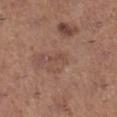Q: Is there a histopathology result?
A: imaged on a skin check; not biopsied
Q: What did automated image analysis measure?
A: a footprint of about 2.5 mm² and two-axis asymmetry of about 0.4; a classifier nevus-likeness of about 0/100 and lesion-presence confidence of about 100/100
Q: What is the lesion's diameter?
A: ≈2.5 mm
Q: What is the imaging modality?
A: ~15 mm tile from a whole-body skin photo
Q: Who is the patient?
A: female, approximately 65 years of age
Q: Where on the body is the lesion?
A: the leg
Q: What lighting was used for the tile?
A: white-light illumination